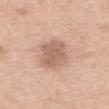Q: Where on the body is the lesion?
A: the upper back
Q: Who is the patient?
A: female, aged 58 to 62
Q: What kind of image is this?
A: ~15 mm tile from a whole-body skin photo